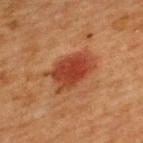{
  "biopsy_status": "not biopsied; imaged during a skin examination",
  "lighting": "cross-polarized",
  "automated_metrics": {
    "area_mm2_approx": 14.0,
    "eccentricity": 0.8,
    "cielab_L": 37,
    "cielab_a": 27,
    "cielab_b": 32,
    "vs_skin_darker_L": 10.0,
    "vs_skin_contrast_norm": 9.0,
    "border_irregularity_0_10": 3.0,
    "color_variation_0_10": 4.0,
    "peripheral_color_asymmetry": 1.0,
    "lesion_detection_confidence_0_100": 100
  },
  "patient": {
    "sex": "female",
    "age_approx": 40
  },
  "image": {
    "source": "total-body photography crop",
    "field_of_view_mm": 15
  },
  "site": "upper back",
  "lesion_size": {
    "long_diameter_mm_approx": 5.5
  }
}